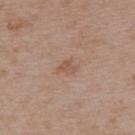| feature | finding |
|---|---|
| notes | no biopsy performed (imaged during a skin exam) |
| subject | female, in their 30s |
| site | the upper back |
| image | ~15 mm tile from a whole-body skin photo |
| tile lighting | white-light |
| size | about 2.5 mm |